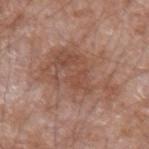Image and clinical context: Automated tile analysis of the lesion measured an area of roughly 31 mm², an outline eccentricity of about 0.8 (0 = round, 1 = elongated), and two-axis asymmetry of about 0.45. It also reported peripheral color asymmetry of about 1.5. From the left forearm. A region of skin cropped from a whole-body photographic capture, roughly 15 mm wide. A male subject, aged approximately 60.